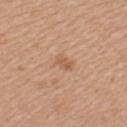| feature | finding |
|---|---|
| follow-up | imaged on a skin check; not biopsied |
| subject | female, aged 33 to 37 |
| site | the left upper arm |
| imaging modality | total-body-photography crop, ~15 mm field of view |